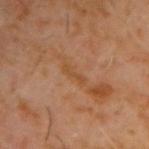follow-up=imaged on a skin check; not biopsied
subject=male, approximately 60 years of age
site=the upper back
image source=~15 mm crop, total-body skin-cancer survey